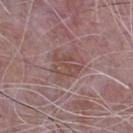Clinical impression: The lesion was photographed on a routine skin check and not biopsied; there is no pathology result.